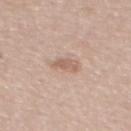workup = catalogued during a skin exam; not biopsied
subject = male, roughly 55 years of age
lesion diameter = ≈3 mm
image-analysis metrics = an eccentricity of roughly 0.75 and two-axis asymmetry of about 0.35; an automated nevus-likeness rating near 25 out of 100 and lesion-presence confidence of about 100/100
image = ~15 mm crop, total-body skin-cancer survey
site = the mid back
illumination = white-light illumination